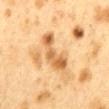Q: Was this lesion biopsied?
A: total-body-photography surveillance lesion; no biopsy
Q: What did automated image analysis measure?
A: a lesion-detection confidence of about 100/100
Q: How large is the lesion?
A: ~5 mm (longest diameter)
Q: Patient demographics?
A: female, aged 38 to 42
Q: What is the anatomic site?
A: the mid back
Q: Illumination type?
A: cross-polarized
Q: How was this image acquired?
A: total-body-photography crop, ~15 mm field of view